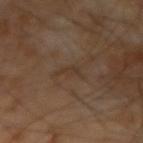{
  "lighting": "cross-polarized",
  "image": {
    "source": "total-body photography crop",
    "field_of_view_mm": 15
  },
  "patient": {
    "sex": "male",
    "age_approx": 65
  },
  "lesion_size": {
    "long_diameter_mm_approx": 3.0
  }
}